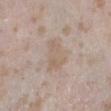* notes — imaged on a skin check; not biopsied
* lighting — white-light
* image source — total-body-photography crop, ~15 mm field of view
* anatomic site — the right lower leg
* lesion diameter — about 4 mm
* patient — female, aged around 25